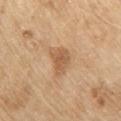<case>
  <biopsy_status>not biopsied; imaged during a skin examination</biopsy_status>
  <automated_metrics>
    <area_mm2_approx>6.5</area_mm2_approx>
    <eccentricity>0.65</eccentricity>
    <cielab_L>58</cielab_L>
    <cielab_a>20</cielab_a>
    <cielab_b>36</cielab_b>
    <vs_skin_contrast_norm>6.5</vs_skin_contrast_norm>
    <color_variation_0_10>2.5</color_variation_0_10>
    <peripheral_color_asymmetry>0.5</peripheral_color_asymmetry>
  </automated_metrics>
  <lesion_size>
    <long_diameter_mm_approx>3.5</long_diameter_mm_approx>
  </lesion_size>
  <image>
    <source>total-body photography crop</source>
    <field_of_view_mm>15</field_of_view_mm>
  </image>
  <patient>
    <sex>male</sex>
    <age_approx>70</age_approx>
  </patient>
  <lighting>white-light</lighting>
  <site>chest</site>
</case>